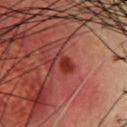A 15 mm close-up extracted from a 3D total-body photography capture.
Located on the chest.
Longest diameter approximately 3 mm.
A male subject, aged around 65.
An algorithmic analysis of the crop reported a mean CIELAB color near L≈35 a*≈32 b*≈29 and roughly 9 lightness units darker than nearby skin. And it measured a border-irregularity index near 5.5/10, a color-variation rating of about 2/10, and peripheral color asymmetry of about 0.5. It also reported an automated nevus-likeness rating near 65 out of 100 and a detector confidence of about 100 out of 100 that the crop contains a lesion.
Imaged with cross-polarized lighting.